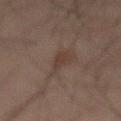Clinical impression:
The lesion was tiled from a total-body skin photograph and was not biopsied.
Context:
The patient is a male in their mid-40s. Cropped from a whole-body photographic skin survey; the tile spans about 15 mm. From the abdomen.